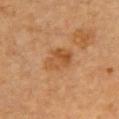Findings:
– notes — total-body-photography surveillance lesion; no biopsy
– subject — male, aged 83 or over
– automated lesion analysis — a border-irregularity rating of about 2/10, a within-lesion color-variation index near 5/10, and peripheral color asymmetry of about 2
– acquisition — ~15 mm tile from a whole-body skin photo
– lesion size — about 3.5 mm
– anatomic site — the chest